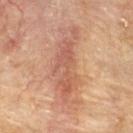Assessment: This lesion was catalogued during total-body skin photography and was not selected for biopsy. Image and clinical context: The lesion is on the upper back. A 15 mm crop from a total-body photograph taken for skin-cancer surveillance. A male subject, aged approximately 85 or older. The lesion-visualizer software estimated a border-irregularity index near 6.5/10, internal color variation of about 5 on a 0–10 scale, and a peripheral color-asymmetry measure near 1.5. And it measured lesion-presence confidence of about 75/100. The tile uses cross-polarized illumination. About 8.5 mm across.